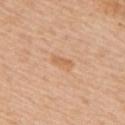The lesion was photographed on a routine skin check and not biopsied; there is no pathology result. Automated image analysis of the tile measured an area of roughly 3.5 mm², an outline eccentricity of about 0.85 (0 = round, 1 = elongated), and a symmetry-axis asymmetry near 0.3. The software also gave a lesion–skin lightness drop of about 7 and a normalized border contrast of about 5. And it measured a border-irregularity index near 3/10 and peripheral color asymmetry of about 1. It also reported a nevus-likeness score of about 0/100. About 3 mm across. Located on the mid back. This is a white-light tile. A close-up tile cropped from a whole-body skin photograph, about 15 mm across. A male patient, aged around 70.